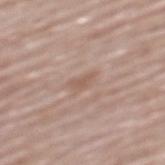- notes: catalogued during a skin exam; not biopsied
- site: the mid back
- image-analysis metrics: a lesion area of about 3.5 mm², a shape eccentricity near 0.8, and a shape-asymmetry score of about 0.4 (0 = symmetric); a classifier nevus-likeness of about 0/100 and a detector confidence of about 100 out of 100 that the crop contains a lesion
- imaging modality: ~15 mm crop, total-body skin-cancer survey
- diameter: about 3 mm
- patient: male, aged 78–82